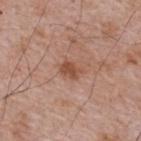No biopsy was performed on this lesion — it was imaged during a full skin examination and was not determined to be concerning. The recorded lesion diameter is about 3 mm. The tile uses white-light illumination. The total-body-photography lesion software estimated an average lesion color of about L≈50 a*≈23 b*≈30 (CIELAB) and a lesion-to-skin contrast of about 7.5 (normalized; higher = more distinct). The analysis additionally found a border-irregularity rating of about 3/10, internal color variation of about 2 on a 0–10 scale, and a peripheral color-asymmetry measure near 0.5. And it measured a classifier nevus-likeness of about 60/100 and a detector confidence of about 100 out of 100 that the crop contains a lesion. A male patient aged around 65. A 15 mm close-up extracted from a 3D total-body photography capture. The lesion is on the back.